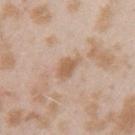The lesion was photographed on a routine skin check and not biopsied; there is no pathology result. This is a white-light tile. The lesion is located on the left upper arm. The subject is a female in their mid- to late 20s. Cropped from a whole-body photographic skin survey; the tile spans about 15 mm.